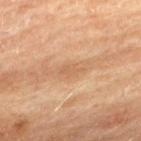Notes:
– location: the upper back
– subject: female, about 80 years old
– diameter: about 2.5 mm
– illumination: cross-polarized illumination
– acquisition: ~15 mm crop, total-body skin-cancer survey